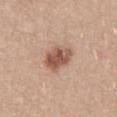Q: Was this lesion biopsied?
A: catalogued during a skin exam; not biopsied
Q: What is the imaging modality?
A: total-body-photography crop, ~15 mm field of view
Q: What is the anatomic site?
A: the lower back
Q: Lesion size?
A: about 4 mm
Q: What are the patient's age and sex?
A: male, aged around 35
Q: How was the tile lit?
A: white-light